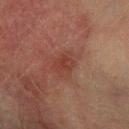Q: Was this lesion biopsied?
A: no biopsy performed (imaged during a skin exam)
Q: Where on the body is the lesion?
A: the right forearm
Q: Patient demographics?
A: male, aged 73 to 77
Q: How was this image acquired?
A: 15 mm crop, total-body photography